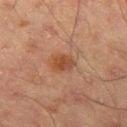Impression:
This lesion was catalogued during total-body skin photography and was not selected for biopsy.
Clinical summary:
A region of skin cropped from a whole-body photographic capture, roughly 15 mm wide. Approximately 3 mm at its widest. Imaged with cross-polarized lighting. A male patient aged approximately 65. Located on the right thigh.